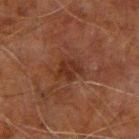Imaged during a routine full-body skin examination; the lesion was not biopsied and no histopathology is available.
A lesion tile, about 15 mm wide, cut from a 3D total-body photograph.
Longest diameter approximately 2.5 mm.
The lesion is on the left arm.
Captured under cross-polarized illumination.
Automated image analysis of the tile measured a footprint of about 4.5 mm², an eccentricity of roughly 0.45, and two-axis asymmetry of about 0.3. The analysis additionally found an average lesion color of about L≈23 a*≈19 b*≈23 (CIELAB), a lesion–skin lightness drop of about 6, and a lesion-to-skin contrast of about 7 (normalized; higher = more distinct).
A male subject aged 58–62.